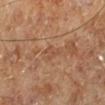Impression:
Recorded during total-body skin imaging; not selected for excision or biopsy.
Background:
Cropped from a whole-body photographic skin survey; the tile spans about 15 mm. The tile uses cross-polarized illumination. About 3 mm across. A male subject, roughly 70 years of age. Located on the left lower leg.